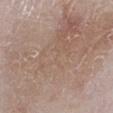{"biopsy_status": "not biopsied; imaged during a skin examination", "lesion_size": {"long_diameter_mm_approx": 18.0}, "lighting": "white-light", "site": "left lower leg", "automated_metrics": {"area_mm2_approx": 145.0, "eccentricity": 0.8, "shape_asymmetry": 0.3, "nevus_likeness_0_100": 0, "lesion_detection_confidence_0_100": 85}, "image": {"source": "total-body photography crop", "field_of_view_mm": 15}, "patient": {"sex": "male", "age_approx": 65}}